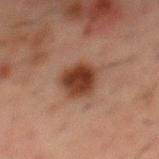Case summary:
• workup — catalogued during a skin exam; not biopsied
• lesion diameter — ≈4 mm
• image source — 15 mm crop, total-body photography
• patient — male, about 50 years old
• location — the mid back
• automated metrics — a lesion area of about 11 mm², an eccentricity of roughly 0.5, and a symmetry-axis asymmetry near 0.2
• lighting — cross-polarized